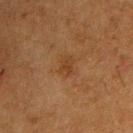Context:
An algorithmic analysis of the crop reported an area of roughly 4.5 mm², an eccentricity of roughly 0.65, and a shape-asymmetry score of about 0.35 (0 = symmetric). And it measured a classifier nevus-likeness of about 15/100. A lesion tile, about 15 mm wide, cut from a 3D total-body photograph. On the upper back. Imaged with cross-polarized lighting. A male subject roughly 75 years of age.